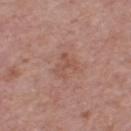This lesion was catalogued during total-body skin photography and was not selected for biopsy.
About 3 mm across.
The subject is a female in their mid-50s.
On the leg.
A close-up tile cropped from a whole-body skin photograph, about 15 mm across.
The tile uses white-light illumination.